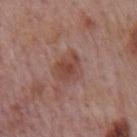<case>
<biopsy_status>not biopsied; imaged during a skin examination</biopsy_status>
<lighting>white-light</lighting>
<site>mid back</site>
<patient>
  <sex>male</sex>
  <age_approx>75</age_approx>
</patient>
<image>
  <source>total-body photography crop</source>
  <field_of_view_mm>15</field_of_view_mm>
</image>
</case>